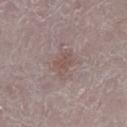The lesion was photographed on a routine skin check and not biopsied; there is no pathology result.
This is a white-light tile.
A female patient, aged around 65.
A roughly 15 mm field-of-view crop from a total-body skin photograph.
From the right lower leg.
About 2.5 mm across.